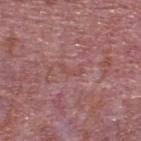This lesion was catalogued during total-body skin photography and was not selected for biopsy.
A 15 mm crop from a total-body photograph taken for skin-cancer surveillance.
Located on the upper back.
A male subject in their mid-70s.